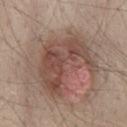Q: Is there a histopathology result?
A: catalogued during a skin exam; not biopsied
Q: How was this image acquired?
A: ~15 mm crop, total-body skin-cancer survey
Q: Where on the body is the lesion?
A: the lower back
Q: How was the tile lit?
A: white-light
Q: Patient demographics?
A: male, aged around 45